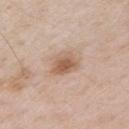Assessment: Captured during whole-body skin photography for melanoma surveillance; the lesion was not biopsied. Acquisition and patient details: On the arm. The subject is a male roughly 55 years of age. Cropped from a total-body skin-imaging series; the visible field is about 15 mm. The tile uses white-light illumination. About 3.5 mm across.